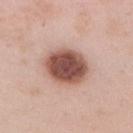image-analysis metrics — a border-irregularity index near 1/10 and a color-variation rating of about 5/10; a classifier nevus-likeness of about 95/100 and a lesion-detection confidence of about 100/100 | image source — ~15 mm tile from a whole-body skin photo | patient — male, in their mid-50s | illumination — white-light illumination | location — the right upper arm.